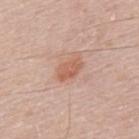Clinical impression:
Captured during whole-body skin photography for melanoma surveillance; the lesion was not biopsied.
Clinical summary:
The total-body-photography lesion software estimated a footprint of about 5 mm², an outline eccentricity of about 0.8 (0 = round, 1 = elongated), and a shape-asymmetry score of about 0.2 (0 = symmetric). It also reported internal color variation of about 3 on a 0–10 scale and a peripheral color-asymmetry measure near 1.5. A male patient, approximately 50 years of age. A lesion tile, about 15 mm wide, cut from a 3D total-body photograph. The lesion is on the mid back. Longest diameter approximately 3.5 mm.